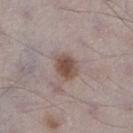No biopsy was performed on this lesion — it was imaged during a full skin examination and was not determined to be concerning. A close-up tile cropped from a whole-body skin photograph, about 15 mm across. The total-body-photography lesion software estimated an area of roughly 7 mm² and a shape eccentricity near 0.6. The software also gave an average lesion color of about L≈49 a*≈16 b*≈22 (CIELAB), about 11 CIELAB-L* units darker than the surrounding skin, and a normalized lesion–skin contrast near 9. Longest diameter approximately 3.5 mm. The patient is a male aged 68–72. Located on the right lower leg.